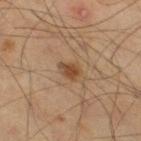Captured during whole-body skin photography for melanoma surveillance; the lesion was not biopsied.
The recorded lesion diameter is about 3 mm.
This image is a 15 mm lesion crop taken from a total-body photograph.
Located on the left thigh.
Automated tile analysis of the lesion measured about 9 CIELAB-L* units darker than the surrounding skin and a lesion-to-skin contrast of about 8.5 (normalized; higher = more distinct). And it measured a nevus-likeness score of about 90/100 and a detector confidence of about 100 out of 100 that the crop contains a lesion.
A male patient, aged approximately 60.